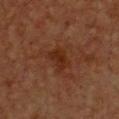The lesion was tiled from a total-body skin photograph and was not biopsied. A female patient, aged 38 to 42. Cropped from a total-body skin-imaging series; the visible field is about 15 mm. From the chest. The lesion's longest dimension is about 3 mm. Captured under cross-polarized illumination.